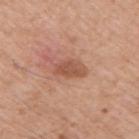notes = total-body-photography surveillance lesion; no biopsy.